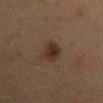Q: Was a biopsy performed?
A: no biopsy performed (imaged during a skin exam)
Q: What are the patient's age and sex?
A: female, in their 50s
Q: What kind of image is this?
A: 15 mm crop, total-body photography
Q: Where on the body is the lesion?
A: the mid back
Q: How large is the lesion?
A: ~3.5 mm (longest diameter)
Q: What lighting was used for the tile?
A: cross-polarized illumination